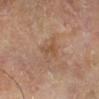| key | value |
|---|---|
| notes | total-body-photography surveillance lesion; no biopsy |
| subject | male, aged around 70 |
| lesion size | ~2.5 mm (longest diameter) |
| lighting | cross-polarized |
| image | ~15 mm crop, total-body skin-cancer survey |
| body site | the right lower leg |